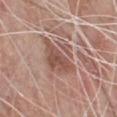No biopsy was performed on this lesion — it was imaged during a full skin examination and was not determined to be concerning.
Longest diameter approximately 5 mm.
A close-up tile cropped from a whole-body skin photograph, about 15 mm across.
A male subject, aged approximately 80.
From the front of the torso.